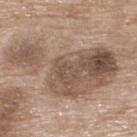Impression: This lesion was catalogued during total-body skin photography and was not selected for biopsy. Image and clinical context: A 15 mm close-up extracted from a 3D total-body photography capture. A female subject about 75 years old. Located on the upper back.